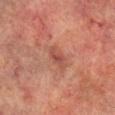The lesion was photographed on a routine skin check and not biopsied; there is no pathology result. A male patient, in their mid-70s. From the right lower leg. This is a cross-polarized tile. Automated tile analysis of the lesion measured an average lesion color of about L≈40 a*≈23 b*≈24 (CIELAB), about 7 CIELAB-L* units darker than the surrounding skin, and a normalized lesion–skin contrast near 6. The software also gave an automated nevus-likeness rating near 0 out of 100. A lesion tile, about 15 mm wide, cut from a 3D total-body photograph.